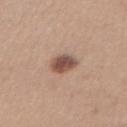No biopsy was performed on this lesion — it was imaged during a full skin examination and was not determined to be concerning.
The lesion is on the abdomen.
A female subject, about 40 years old.
Imaged with white-light lighting.
Longest diameter approximately 3 mm.
A region of skin cropped from a whole-body photographic capture, roughly 15 mm wide.